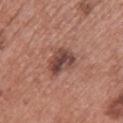Findings:
• notes — catalogued during a skin exam; not biopsied
• lesion diameter — ~3.5 mm (longest diameter)
• site — the upper back
• subject — female, about 60 years old
• lighting — white-light illumination
• acquisition — ~15 mm tile from a whole-body skin photo